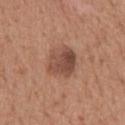Impression: No biopsy was performed on this lesion — it was imaged during a full skin examination and was not determined to be concerning. Clinical summary: A female subject roughly 70 years of age. Automated image analysis of the tile measured a mean CIELAB color near L≈48 a*≈22 b*≈27 and roughly 11 lightness units darker than nearby skin. Captured under white-light illumination. From the upper back. Cropped from a total-body skin-imaging series; the visible field is about 15 mm.